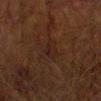site: the right upper arm
acquisition: ~15 mm tile from a whole-body skin photo
lesion diameter: about 4 mm
automated lesion analysis: a mean CIELAB color near L≈18 a*≈15 b*≈19, about 3 CIELAB-L* units darker than the surrounding skin, and a lesion-to-skin contrast of about 5 (normalized; higher = more distinct); a nevus-likeness score of about 0/100 and a lesion-detection confidence of about 95/100
patient: male, approximately 75 years of age